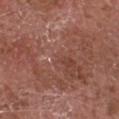Case summary:
- biopsy status — total-body-photography surveillance lesion; no biopsy
- subject — male, about 75 years old
- image-analysis metrics — an area of roughly 49 mm²; an average lesion color of about L≈45 a*≈23 b*≈26 (CIELAB), about 6 CIELAB-L* units darker than the surrounding skin, and a lesion-to-skin contrast of about 4.5 (normalized; higher = more distinct); a border-irregularity rating of about 10/10, a within-lesion color-variation index near 4/10, and radial color variation of about 1.5
- image source — 15 mm crop, total-body photography
- body site — the head or neck
- size — ≈16 mm
- illumination — white-light